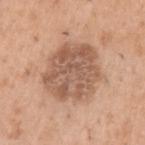Imaged during a routine full-body skin examination; the lesion was not biopsied and no histopathology is available.
The lesion is located on the left upper arm.
Cropped from a total-body skin-imaging series; the visible field is about 15 mm.
A male subject, in their 50s.